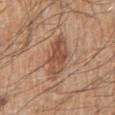follow-up — imaged on a skin check; not biopsied | subject — male, aged approximately 80 | lesion size — ~6 mm (longest diameter) | image source — ~15 mm tile from a whole-body skin photo | automated lesion analysis — two-axis asymmetry of about 0.25; a lesion color around L≈51 a*≈21 b*≈31 in CIELAB, a lesion–skin lightness drop of about 11, and a lesion-to-skin contrast of about 7.5 (normalized; higher = more distinct); a classifier nevus-likeness of about 20/100 and lesion-presence confidence of about 100/100 | site — the right upper arm.